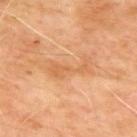Part of a total-body skin-imaging series; this lesion was reviewed on a skin check and was not flagged for biopsy. About 5 mm across. An algorithmic analysis of the crop reported border irregularity of about 8 on a 0–10 scale and peripheral color asymmetry of about 0.5. The software also gave a nevus-likeness score of about 0/100 and a lesion-detection confidence of about 100/100. The lesion is on the upper back. A male subject aged 63 to 67. Captured under cross-polarized illumination. Cropped from a whole-body photographic skin survey; the tile spans about 15 mm.